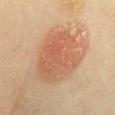Q: What kind of image is this?
A: 15 mm crop, total-body photography
Q: Patient demographics?
A: female, about 60 years old
Q: What is the lesion's diameter?
A: ≈7.5 mm
Q: Illumination type?
A: cross-polarized
Q: What is the anatomic site?
A: the front of the torso